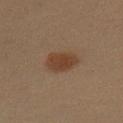notes: catalogued during a skin exam; not biopsied | patient: female, aged approximately 40 | image source: ~15 mm tile from a whole-body skin photo | body site: the left upper arm.